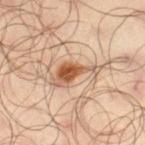  lesion_size:
    long_diameter_mm_approx: 6.5
  patient:
    sex: male
    age_approx: 65
  lighting: cross-polarized
  site: left thigh
  image:
    source: total-body photography crop
    field_of_view_mm: 15